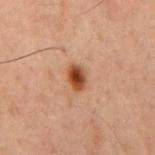No biopsy was performed on this lesion — it was imaged during a full skin examination and was not determined to be concerning. The tile uses cross-polarized illumination. Measured at roughly 3 mm in maximum diameter. An algorithmic analysis of the crop reported an outline eccentricity of about 0.7 (0 = round, 1 = elongated). The software also gave a lesion color around L≈36 a*≈21 b*≈28 in CIELAB, roughly 13 lightness units darker than nearby skin, and a normalized lesion–skin contrast near 11. The analysis additionally found an automated nevus-likeness rating near 100 out of 100 and lesion-presence confidence of about 100/100. The subject is a male approximately 60 years of age. A region of skin cropped from a whole-body photographic capture, roughly 15 mm wide. The lesion is on the back.